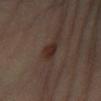The lesion was photographed on a routine skin check and not biopsied; there is no pathology result. Longest diameter approximately 3 mm. The tile uses cross-polarized illumination. A female patient in their 60s. Cropped from a total-body skin-imaging series; the visible field is about 15 mm. From the left leg.